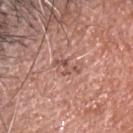Case summary:
* follow-up: imaged on a skin check; not biopsied
* patient: female, aged approximately 50
* body site: the head or neck
* image source: ~15 mm tile from a whole-body skin photo